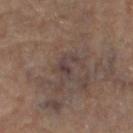Captured during whole-body skin photography for melanoma surveillance; the lesion was not biopsied.
A 15 mm crop from a total-body photograph taken for skin-cancer surveillance.
Longest diameter approximately 3.5 mm.
A male subject in their mid- to late 80s or older.
From the leg.
Captured under cross-polarized illumination.